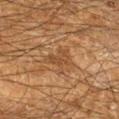follow-up — imaged on a skin check; not biopsied | size — ≈2.5 mm | image — total-body-photography crop, ~15 mm field of view | site — the right lower leg | illumination — cross-polarized | patient — male, in their 60s.